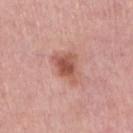Imaged during a routine full-body skin examination; the lesion was not biopsied and no histopathology is available. Automated tile analysis of the lesion measured a shape eccentricity near 0.7 and a symmetry-axis asymmetry near 0.4. The analysis additionally found a mean CIELAB color near L≈55 a*≈26 b*≈28, a lesion–skin lightness drop of about 12, and a normalized border contrast of about 8.5. And it measured a border-irregularity rating of about 4.5/10, internal color variation of about 4 on a 0–10 scale, and radial color variation of about 1. The analysis additionally found a classifier nevus-likeness of about 80/100 and a detector confidence of about 100 out of 100 that the crop contains a lesion. Longest diameter approximately 4 mm. The lesion is on the right upper arm. The subject is a female in their mid- to late 60s. Captured under white-light illumination. A region of skin cropped from a whole-body photographic capture, roughly 15 mm wide.